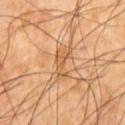The recorded lesion diameter is about 4 mm.
A male patient in their mid-60s.
Located on the front of the torso.
Cropped from a whole-body photographic skin survey; the tile spans about 15 mm.
An algorithmic analysis of the crop reported a lesion area of about 5.5 mm², an outline eccentricity of about 0.9 (0 = round, 1 = elongated), and a shape-asymmetry score of about 0.4 (0 = symmetric). The software also gave an average lesion color of about L≈56 a*≈21 b*≈38 (CIELAB) and roughly 8 lightness units darker than nearby skin. The analysis additionally found border irregularity of about 5 on a 0–10 scale, a color-variation rating of about 2/10, and radial color variation of about 0.5.
This is a cross-polarized tile.